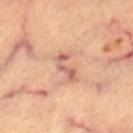location: the left thigh | lesion size: about 3.5 mm | lighting: cross-polarized illumination | image source: total-body-photography crop, ~15 mm field of view | subject: aged 58 to 62.